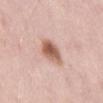The lesion is located on the abdomen.
The recorded lesion diameter is about 3.5 mm.
Cropped from a whole-body photographic skin survey; the tile spans about 15 mm.
A male subject roughly 55 years of age.
This is a white-light tile.